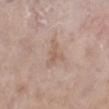Clinical impression:
The lesion was photographed on a routine skin check and not biopsied; there is no pathology result.
Clinical summary:
Longest diameter approximately 4 mm. The subject is a female about 75 years old. The tile uses white-light illumination. The lesion is located on the right lower leg. A lesion tile, about 15 mm wide, cut from a 3D total-body photograph.